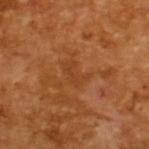Imaged during a routine full-body skin examination; the lesion was not biopsied and no histopathology is available.
Approximately 3 mm at its widest.
This is a cross-polarized tile.
Automated tile analysis of the lesion measured an area of roughly 4 mm², an eccentricity of roughly 0.8, and a symmetry-axis asymmetry near 0.4. The software also gave a border-irregularity index near 5/10, internal color variation of about 1 on a 0–10 scale, and a peripheral color-asymmetry measure near 0.
A male patient in their mid-60s.
Cropped from a total-body skin-imaging series; the visible field is about 15 mm.